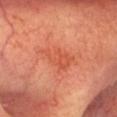A roughly 15 mm field-of-view crop from a total-body skin photograph.
The patient is a male aged around 65.
Located on the head or neck.